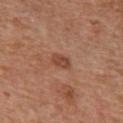Captured during whole-body skin photography for melanoma surveillance; the lesion was not biopsied. This is a white-light tile. A 15 mm close-up extracted from a 3D total-body photography capture. Measured at roughly 2.5 mm in maximum diameter. The subject is a female aged 73 to 77. From the chest. The total-body-photography lesion software estimated a footprint of about 4 mm², a shape eccentricity near 0.75, and two-axis asymmetry of about 0.2. The analysis additionally found an automated nevus-likeness rating near 75 out of 100.